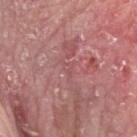The lesion is on the head or neck.
A 15 mm close-up extracted from a 3D total-body photography capture.
The tile uses white-light illumination.
The patient is a male in their mid-60s.
About 1.5 mm across.
Histopathology of the biopsied lesion showed a verruca.